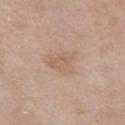Captured during whole-body skin photography for melanoma surveillance; the lesion was not biopsied. From the chest. The total-body-photography lesion software estimated an area of roughly 9 mm². The analysis additionally found a mean CIELAB color near L≈61 a*≈17 b*≈29, about 6 CIELAB-L* units darker than the surrounding skin, and a normalized lesion–skin contrast near 4.5. It also reported border irregularity of about 3.5 on a 0–10 scale and peripheral color asymmetry of about 0.5. The software also gave lesion-presence confidence of about 100/100. A region of skin cropped from a whole-body photographic capture, roughly 15 mm wide. The patient is a female in their 40s. About 4 mm across. This is a white-light tile.